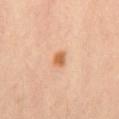Impression: This lesion was catalogued during total-body skin photography and was not selected for biopsy.